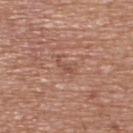biopsy status: imaged on a skin check; not biopsied
patient: male, aged approximately 65
TBP lesion metrics: a lesion area of about 3.5 mm² and a symmetry-axis asymmetry near 0.5; a lesion color around L≈51 a*≈22 b*≈27 in CIELAB and roughly 7 lightness units darker than nearby skin; a border-irregularity index near 6/10, a within-lesion color-variation index near 1.5/10, and peripheral color asymmetry of about 0.5
acquisition: ~15 mm tile from a whole-body skin photo
body site: the upper back
size: about 3 mm
lighting: white-light illumination